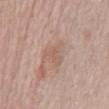This lesion was catalogued during total-body skin photography and was not selected for biopsy. The lesion is on the abdomen. A male subject about 70 years old. Cropped from a whole-body photographic skin survey; the tile spans about 15 mm. About 4 mm across.